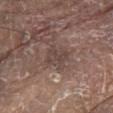Assessment:
Captured during whole-body skin photography for melanoma surveillance; the lesion was not biopsied.
Background:
A 15 mm close-up tile from a total-body photography series done for melanoma screening. The lesion is on the abdomen. A male patient, aged 78 to 82.